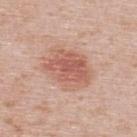Captured during whole-body skin photography for melanoma surveillance; the lesion was not biopsied.
The lesion is located on the upper back.
The patient is a male in their 40s.
A 15 mm crop from a total-body photograph taken for skin-cancer surveillance.